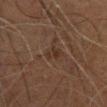Context: Located on the front of the torso. A 15 mm crop from a total-body photograph taken for skin-cancer surveillance. The subject is a male aged around 45.